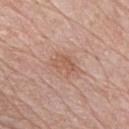Context:
The subject is a male aged 78 to 82. The tile uses white-light illumination. A 15 mm close-up extracted from a 3D total-body photography capture. Longest diameter approximately 3 mm. The lesion is on the chest.